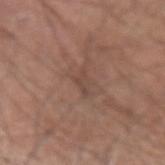Image and clinical context: Located on the left forearm. This image is a 15 mm lesion crop taken from a total-body photograph. The patient is a male roughly 55 years of age. Measured at roughly 3 mm in maximum diameter. Automated image analysis of the tile measured a footprint of about 2.5 mm² and a shape-asymmetry score of about 0.5 (0 = symmetric). And it measured a lesion–skin lightness drop of about 6. The analysis additionally found border irregularity of about 5.5 on a 0–10 scale, internal color variation of about 0 on a 0–10 scale, and peripheral color asymmetry of about 0. It also reported a nevus-likeness score of about 0/100 and lesion-presence confidence of about 65/100. This is a white-light tile.